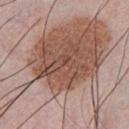<tbp_lesion>
  <automated_metrics>
    <border_irregularity_0_10>9.0</border_irregularity_0_10>
    <color_variation_0_10>5.0</color_variation_0_10>
    <peripheral_color_asymmetry>2.0</peripheral_color_asymmetry>
  </automated_metrics>
  <patient>
    <sex>male</sex>
    <age_approx>35</age_approx>
  </patient>
  <lesion_size>
    <long_diameter_mm_approx>6.0</long_diameter_mm_approx>
  </lesion_size>
  <site>chest</site>
  <lighting>white-light</lighting>
  <image>
    <source>total-body photography crop</source>
    <field_of_view_mm>15</field_of_view_mm>
  </image>
</tbp_lesion>